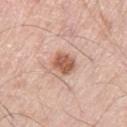image source = 15 mm crop, total-body photography; anatomic site = the leg; subject = male, aged approximately 80.